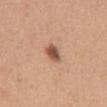Recorded during total-body skin imaging; not selected for excision or biopsy. The lesion is located on the abdomen. The subject is a female aged approximately 30. Approximately 2.5 mm at its widest. A close-up tile cropped from a whole-body skin photograph, about 15 mm across.